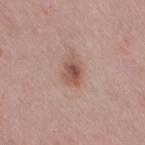notes: imaged on a skin check; not biopsied
lesion diameter: ≈3 mm
patient: female, aged around 40
site: the right thigh
acquisition: 15 mm crop, total-body photography